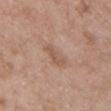Context: Located on the chest. A region of skin cropped from a whole-body photographic capture, roughly 15 mm wide. This is a white-light tile. A male patient in their 50s. About 3.5 mm across.